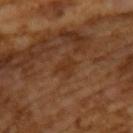- subject: male, about 65 years old
- tile lighting: cross-polarized illumination
- image: 15 mm crop, total-body photography